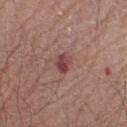{"biopsy_status": "not biopsied; imaged during a skin examination", "site": "right forearm", "lighting": "white-light", "lesion_size": {"long_diameter_mm_approx": 2.5}, "image": {"source": "total-body photography crop", "field_of_view_mm": 15}, "automated_metrics": {"cielab_L": 43, "cielab_a": 25, "cielab_b": 19, "vs_skin_darker_L": 11.0, "vs_skin_contrast_norm": 8.5, "border_irregularity_0_10": 2.5, "peripheral_color_asymmetry": 1.5}, "patient": {"sex": "male", "age_approx": 65}}